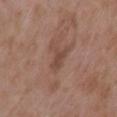Q: Lesion location?
A: the left upper arm
Q: What are the patient's age and sex?
A: female, in their mid-30s
Q: Lesion size?
A: ≈3 mm
Q: How was the tile lit?
A: white-light
Q: What kind of image is this?
A: total-body-photography crop, ~15 mm field of view
Q: What did automated image analysis measure?
A: a footprint of about 3 mm² and an outline eccentricity of about 0.85 (0 = round, 1 = elongated); an average lesion color of about L≈45 a*≈19 b*≈26 (CIELAB) and a lesion-to-skin contrast of about 6 (normalized; higher = more distinct); border irregularity of about 4 on a 0–10 scale and a peripheral color-asymmetry measure near 0